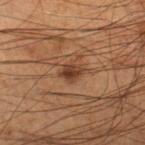workup: imaged on a skin check; not biopsied
image source: 15 mm crop, total-body photography
lighting: cross-polarized
automated lesion analysis: an area of roughly 5 mm² and an eccentricity of roughly 0.7; a nevus-likeness score of about 90/100
body site: the right lower leg
subject: male, aged 53 to 57
size: ≈3 mm